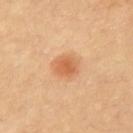workup: no biopsy performed (imaged during a skin exam) | image-analysis metrics: a lesion area of about 7 mm² | tile lighting: cross-polarized | location: the chest | lesion size: ~3 mm (longest diameter) | image: total-body-photography crop, ~15 mm field of view.